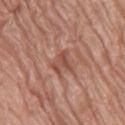Located on the right thigh.
Longest diameter approximately 2.5 mm.
A 15 mm crop from a total-body photograph taken for skin-cancer surveillance.
A female patient aged around 75.
Captured under white-light illumination.